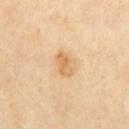Case summary:
- biopsy status · catalogued during a skin exam; not biopsied
- acquisition · ~15 mm tile from a whole-body skin photo
- anatomic site · the chest